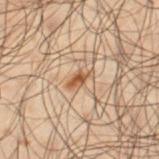follow-up: no biopsy performed (imaged during a skin exam)
automated lesion analysis: an eccentricity of roughly 0.85 and two-axis asymmetry of about 0.3; a lesion color around L≈42 a*≈16 b*≈29 in CIELAB, roughly 10 lightness units darker than nearby skin, and a lesion-to-skin contrast of about 9 (normalized; higher = more distinct); a color-variation rating of about 2.5/10 and a peripheral color-asymmetry measure near 1; an automated nevus-likeness rating near 95 out of 100
body site: the left thigh
patient: male, roughly 50 years of age
acquisition: ~15 mm crop, total-body skin-cancer survey
tile lighting: cross-polarized illumination
lesion diameter: about 3 mm